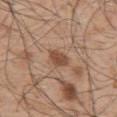{"biopsy_status": "not biopsied; imaged during a skin examination", "patient": {"sex": "male", "age_approx": 50}, "automated_metrics": {"area_mm2_approx": 4.0, "eccentricity": 0.85, "nevus_likeness_0_100": 80, "lesion_detection_confidence_0_100": 100}, "lighting": "white-light", "lesion_size": {"long_diameter_mm_approx": 3.0}, "image": {"source": "total-body photography crop", "field_of_view_mm": 15}, "site": "upper back"}